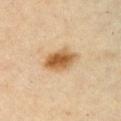Clinical impression:
Part of a total-body skin-imaging series; this lesion was reviewed on a skin check and was not flagged for biopsy.
Acquisition and patient details:
Longest diameter approximately 4 mm. The subject is a female about 45 years old. On the left upper arm. The total-body-photography lesion software estimated a lesion–skin lightness drop of about 12 and a lesion-to-skin contrast of about 10 (normalized; higher = more distinct). The analysis additionally found a border-irregularity rating of about 1.5/10, a within-lesion color-variation index near 5.5/10, and peripheral color asymmetry of about 1.5. A roughly 15 mm field-of-view crop from a total-body skin photograph. The tile uses cross-polarized illumination.